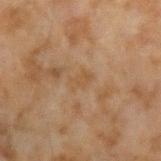Imaged during a routine full-body skin examination; the lesion was not biopsied and no histopathology is available.
About 2.5 mm across.
This image is a 15 mm lesion crop taken from a total-body photograph.
A male patient about 45 years old.
Located on the left forearm.